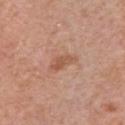image:
  source: total-body photography crop
  field_of_view_mm: 15
patient:
  sex: male
  age_approx: 70
site: chest
automated_metrics:
  area_mm2_approx: 4.5
  eccentricity: 0.9
  shape_asymmetry: 0.35
  peripheral_color_asymmetry: 1.0
lesion_size:
  long_diameter_mm_approx: 3.5